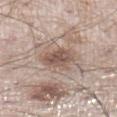biopsy status = imaged on a skin check; not biopsied | diameter = ≈3.5 mm | acquisition = 15 mm crop, total-body photography | site = the left lower leg | patient = male, aged approximately 60.